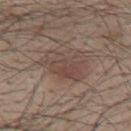{"biopsy_status": "not biopsied; imaged during a skin examination", "site": "mid back", "patient": {"sex": "male", "age_approx": 45}, "lighting": "white-light", "image": {"source": "total-body photography crop", "field_of_view_mm": 15}}